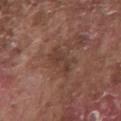Assessment: The lesion was photographed on a routine skin check and not biopsied; there is no pathology result. Context: From the chest. Measured at roughly 4 mm in maximum diameter. A male subject, aged 73–77. A lesion tile, about 15 mm wide, cut from a 3D total-body photograph. This is a white-light tile.